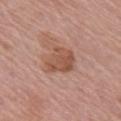biopsy_status: not biopsied; imaged during a skin examination
lesion_size:
  long_diameter_mm_approx: 4.0
lighting: white-light
site: arm
automated_metrics:
  area_mm2_approx: 10.0
  shape_asymmetry: 0.3
  vs_skin_darker_L: 9.0
  vs_skin_contrast_norm: 7.0
  border_irregularity_0_10: 2.5
  nevus_likeness_0_100: 5
  lesion_detection_confidence_0_100: 100
image:
  source: total-body photography crop
  field_of_view_mm: 15
patient:
  sex: female
  age_approx: 60